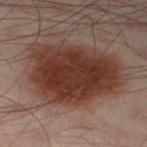Captured during whole-body skin photography for melanoma surveillance; the lesion was not biopsied. A male patient aged 48 to 52. The recorded lesion diameter is about 9.5 mm. The lesion is located on the left thigh. A 15 mm close-up tile from a total-body photography series done for melanoma screening. The tile uses cross-polarized illumination.